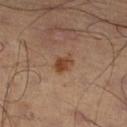The lesion was photographed on a routine skin check and not biopsied; there is no pathology result. A male subject in their 50s. Approximately 3 mm at its widest. Located on the left leg. An algorithmic analysis of the crop reported a lesion area of about 4 mm², an outline eccentricity of about 0.75 (0 = round, 1 = elongated), and a symmetry-axis asymmetry near 0.25. The analysis additionally found a lesion–skin lightness drop of about 10 and a normalized border contrast of about 8.5. And it measured a border-irregularity rating of about 2.5/10 and a color-variation rating of about 2/10. The software also gave a classifier nevus-likeness of about 90/100 and a lesion-detection confidence of about 100/100. This is a cross-polarized tile. This image is a 15 mm lesion crop taken from a total-body photograph.